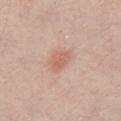Assessment: Recorded during total-body skin imaging; not selected for excision or biopsy. Image and clinical context: The patient is a male aged 28 to 32. The lesion-visualizer software estimated an outline eccentricity of about 0.7 (0 = round, 1 = elongated). It also reported a border-irregularity index near 2/10 and radial color variation of about 1. The analysis additionally found an automated nevus-likeness rating near 30 out of 100 and a detector confidence of about 100 out of 100 that the crop contains a lesion. Imaged with white-light lighting. Cropped from a total-body skin-imaging series; the visible field is about 15 mm. The recorded lesion diameter is about 3 mm.